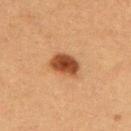Case summary:
* notes — imaged on a skin check; not biopsied
* anatomic site — the upper back
* image source — ~15 mm crop, total-body skin-cancer survey
* lesion diameter — ~4 mm (longest diameter)
* patient — female, aged 38 to 42
* TBP lesion metrics — a nevus-likeness score of about 100/100 and lesion-presence confidence of about 100/100
* tile lighting — cross-polarized